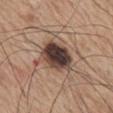{"biopsy_status": "not biopsied; imaged during a skin examination", "lighting": "white-light", "site": "mid back", "patient": {"sex": "male", "age_approx": 75}, "lesion_size": {"long_diameter_mm_approx": 5.0}, "image": {"source": "total-body photography crop", "field_of_view_mm": 15}}